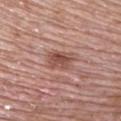notes: imaged on a skin check; not biopsied | image: ~15 mm tile from a whole-body skin photo | diameter: about 3 mm | location: the upper back | tile lighting: white-light illumination | subject: male, about 70 years old | TBP lesion metrics: an outline eccentricity of about 0.5 (0 = round, 1 = elongated).